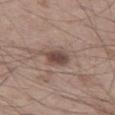Captured during whole-body skin photography for melanoma surveillance; the lesion was not biopsied. On the left thigh. A male patient in their 50s. Automated tile analysis of the lesion measured a within-lesion color-variation index near 3/10 and a peripheral color-asymmetry measure near 1. It also reported a nevus-likeness score of about 80/100 and lesion-presence confidence of about 100/100. Measured at roughly 3.5 mm in maximum diameter. A 15 mm crop from a total-body photograph taken for skin-cancer surveillance.